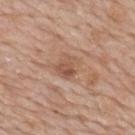<tbp_lesion>
  <image>
    <source>total-body photography crop</source>
    <field_of_view_mm>15</field_of_view_mm>
  </image>
  <site>mid back</site>
  <patient>
    <sex>male</sex>
    <age_approx>60</age_approx>
  </patient>
</tbp_lesion>